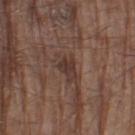The lesion was photographed on a routine skin check and not biopsied; there is no pathology result.
The patient is a male aged approximately 80.
Automated tile analysis of the lesion measured an area of roughly 6 mm², an outline eccentricity of about 0.8 (0 = round, 1 = elongated), and two-axis asymmetry of about 0.4. And it measured an average lesion color of about L≈36 a*≈17 b*≈22 (CIELAB), roughly 8 lightness units darker than nearby skin, and a normalized lesion–skin contrast near 7.5. The analysis additionally found a border-irregularity index near 5/10, a color-variation rating of about 2/10, and peripheral color asymmetry of about 0.5. It also reported an automated nevus-likeness rating near 0 out of 100 and a lesion-detection confidence of about 90/100.
The recorded lesion diameter is about 3.5 mm.
The lesion is located on the left thigh.
A lesion tile, about 15 mm wide, cut from a 3D total-body photograph.
This is a white-light tile.